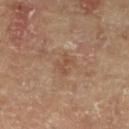Findings:
* notes: total-body-photography surveillance lesion; no biopsy
* anatomic site: the right lower leg
* patient: female, aged approximately 75
* lesion size: about 2.5 mm
* lighting: cross-polarized
* imaging modality: ~15 mm tile from a whole-body skin photo